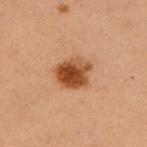Captured during whole-body skin photography for melanoma surveillance; the lesion was not biopsied. Imaged with cross-polarized lighting. From the left upper arm. A female patient in their 50s. This image is a 15 mm lesion crop taken from a total-body photograph.